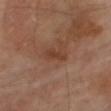On the right thigh. A male patient, aged 68 to 72. The recorded lesion diameter is about 2.5 mm. Imaged with cross-polarized lighting. An algorithmic analysis of the crop reported a footprint of about 3 mm², an eccentricity of roughly 0.9, and a symmetry-axis asymmetry near 0.35. The software also gave a mean CIELAB color near L≈38 a*≈21 b*≈29, a lesion–skin lightness drop of about 7, and a lesion-to-skin contrast of about 6.5 (normalized; higher = more distinct). The analysis additionally found a within-lesion color-variation index near 0.5/10 and peripheral color asymmetry of about 0. A lesion tile, about 15 mm wide, cut from a 3D total-body photograph.